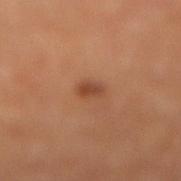The lesion was tiled from a total-body skin photograph and was not biopsied. Imaged with cross-polarized lighting. Measured at roughly 2 mm in maximum diameter. The lesion is on the leg. A male patient approximately 65 years of age. Cropped from a total-body skin-imaging series; the visible field is about 15 mm.